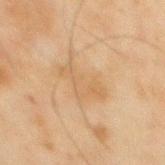| feature | finding |
|---|---|
| follow-up | catalogued during a skin exam; not biopsied |
| lesion size | about 5.5 mm |
| lighting | cross-polarized illumination |
| acquisition | ~15 mm tile from a whole-body skin photo |
| site | the mid back |
| TBP lesion metrics | an area of roughly 9.5 mm², an eccentricity of roughly 0.85, and a shape-asymmetry score of about 0.5 (0 = symmetric); peripheral color asymmetry of about 0.5 |
| patient | male, roughly 45 years of age |